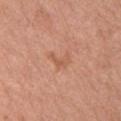No biopsy was performed on this lesion — it was imaged during a full skin examination and was not determined to be concerning. Located on the chest. A 15 mm crop from a total-body photograph taken for skin-cancer surveillance. The patient is a female roughly 65 years of age.